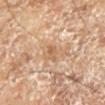Q: Was a biopsy performed?
A: imaged on a skin check; not biopsied
Q: How was the tile lit?
A: cross-polarized
Q: Lesion location?
A: the left lower leg
Q: How was this image acquired?
A: 15 mm crop, total-body photography
Q: What are the patient's age and sex?
A: male, in their 70s